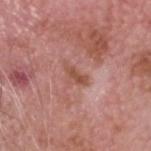Case summary:
– follow-up · imaged on a skin check; not biopsied
– lighting · white-light
– image · ~15 mm crop, total-body skin-cancer survey
– subject · male, roughly 75 years of age
– location · the head or neck
– size · about 3 mm
– TBP lesion metrics · an average lesion color of about L≈52 a*≈24 b*≈28 (CIELAB), about 9 CIELAB-L* units darker than the surrounding skin, and a normalized border contrast of about 7; a classifier nevus-likeness of about 0/100 and a detector confidence of about 100 out of 100 that the crop contains a lesion